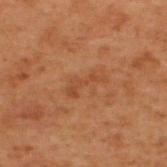notes: catalogued during a skin exam; not biopsied | tile lighting: cross-polarized illumination | TBP lesion metrics: a lesion area of about 4.5 mm², a shape eccentricity near 0.95, and a shape-asymmetry score of about 0.6 (0 = symmetric); roughly 5 lightness units darker than nearby skin and a lesion-to-skin contrast of about 5 (normalized; higher = more distinct) | site: the upper back | lesion diameter: about 4 mm | imaging modality: total-body-photography crop, ~15 mm field of view | subject: male, approximately 70 years of age.